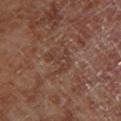Q: Was this lesion biopsied?
A: total-body-photography surveillance lesion; no biopsy
Q: Illumination type?
A: cross-polarized illumination
Q: How was this image acquired?
A: total-body-photography crop, ~15 mm field of view
Q: Automated lesion metrics?
A: a footprint of about 5 mm², an outline eccentricity of about 0.75 (0 = round, 1 = elongated), and a shape-asymmetry score of about 0.55 (0 = symmetric); a classifier nevus-likeness of about 0/100 and lesion-presence confidence of about 90/100
Q: What is the anatomic site?
A: the right lower leg
Q: How large is the lesion?
A: about 3.5 mm
Q: Who is the patient?
A: male, aged 68–72